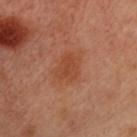biopsy status=no biopsy performed (imaged during a skin exam)
image-analysis metrics=a lesion–skin lightness drop of about 6 and a normalized lesion–skin contrast near 6; border irregularity of about 2.5 on a 0–10 scale and a color-variation rating of about 2/10; a classifier nevus-likeness of about 0/100 and a lesion-detection confidence of about 100/100
lesion diameter=~3 mm (longest diameter)
image=~15 mm crop, total-body skin-cancer survey
location=the chest
illumination=cross-polarized illumination
patient=female, about 55 years old